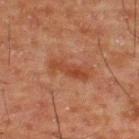| key | value |
|---|---|
| tile lighting | cross-polarized |
| diameter | ≈5 mm |
| subject | male, about 50 years old |
| imaging modality | 15 mm crop, total-body photography |
| site | the upper back |
| TBP lesion metrics | a footprint of about 7.5 mm², an outline eccentricity of about 0.95 (0 = round, 1 = elongated), and two-axis asymmetry of about 0.25; internal color variation of about 3 on a 0–10 scale; an automated nevus-likeness rating near 55 out of 100 and a detector confidence of about 100 out of 100 that the crop contains a lesion |